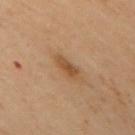Impression:
The lesion was tiled from a total-body skin photograph and was not biopsied.
Image and clinical context:
A region of skin cropped from a whole-body photographic capture, roughly 15 mm wide. The lesion's longest dimension is about 2.5 mm. On the upper back. The total-body-photography lesion software estimated roughly 8 lightness units darker than nearby skin. It also reported a border-irregularity index near 2/10, a within-lesion color-variation index near 2/10, and peripheral color asymmetry of about 0.5. The patient is a female aged approximately 60.